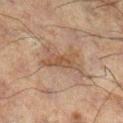Part of a total-body skin-imaging series; this lesion was reviewed on a skin check and was not flagged for biopsy.